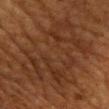<record>
<biopsy_status>not biopsied; imaged during a skin examination</biopsy_status>
<image>
  <source>total-body photography crop</source>
  <field_of_view_mm>15</field_of_view_mm>
</image>
<patient>
  <sex>male</sex>
  <age_approx>60</age_approx>
</patient>
<site>front of the torso</site>
</record>